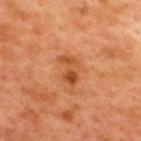No biopsy was performed on this lesion — it was imaged during a full skin examination and was not determined to be concerning. Approximately 4 mm at its widest. On the back. A roughly 15 mm field-of-view crop from a total-body skin photograph. Automated image analysis of the tile measured an area of roughly 6 mm², an outline eccentricity of about 0.85 (0 = round, 1 = elongated), and a symmetry-axis asymmetry near 0.4. And it measured roughly 10 lightness units darker than nearby skin and a lesion-to-skin contrast of about 7 (normalized; higher = more distinct). The analysis additionally found an automated nevus-likeness rating near 30 out of 100 and lesion-presence confidence of about 100/100. The subject is a male approximately 50 years of age. This is a cross-polarized tile.